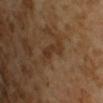| field | value |
|---|---|
| notes | imaged on a skin check; not biopsied |